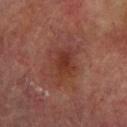Findings:
* follow-up · total-body-photography surveillance lesion; no biopsy
* size · ~5 mm (longest diameter)
* image · ~15 mm crop, total-body skin-cancer survey
* subject · male, aged 73–77
* site · the arm
* lighting · cross-polarized